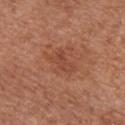Q: Is there a histopathology result?
A: imaged on a skin check; not biopsied
Q: Patient demographics?
A: female, about 80 years old
Q: How was this image acquired?
A: ~15 mm crop, total-body skin-cancer survey
Q: Lesion size?
A: ~3 mm (longest diameter)
Q: What lighting was used for the tile?
A: white-light
Q: What is the anatomic site?
A: the left upper arm